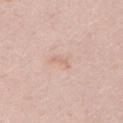Clinical impression: Imaged during a routine full-body skin examination; the lesion was not biopsied and no histopathology is available. Image and clinical context: A 15 mm close-up tile from a total-body photography series done for melanoma screening. This is a white-light tile. The lesion is located on the left upper arm. Measured at roughly 2.5 mm in maximum diameter. The patient is a male aged around 50.